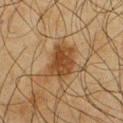Impression: The lesion was photographed on a routine skin check and not biopsied; there is no pathology result. Acquisition and patient details: The lesion's longest dimension is about 4 mm. Cropped from a whole-body photographic skin survey; the tile spans about 15 mm. Automated image analysis of the tile measured a lesion color around L≈36 a*≈18 b*≈32 in CIELAB and roughly 10 lightness units darker than nearby skin. It also reported a classifier nevus-likeness of about 90/100 and a lesion-detection confidence of about 100/100. A male subject aged 63–67. The lesion is on the front of the torso.